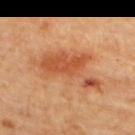notes: imaged on a skin check; not biopsied
acquisition: total-body-photography crop, ~15 mm field of view
automated metrics: an area of roughly 20 mm², a shape eccentricity near 0.85, and a shape-asymmetry score of about 0.6 (0 = symmetric); a lesion color around L≈53 a*≈28 b*≈38 in CIELAB, roughly 10 lightness units darker than nearby skin, and a normalized border contrast of about 7; a classifier nevus-likeness of about 80/100
anatomic site: the left arm
illumination: cross-polarized
patient: female, roughly 65 years of age
lesion size: ≈8.5 mm